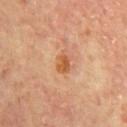notes — imaged on a skin check; not biopsied
site — the chest
patient — male, about 65 years old
imaging modality — 15 mm crop, total-body photography
tile lighting — cross-polarized
TBP lesion metrics — border irregularity of about 2.5 on a 0–10 scale, internal color variation of about 3 on a 0–10 scale, and peripheral color asymmetry of about 1